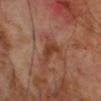<lesion>
  <biopsy_status>not biopsied; imaged during a skin examination</biopsy_status>
  <lesion_size>
    <long_diameter_mm_approx>3.5</long_diameter_mm_approx>
  </lesion_size>
  <image>
    <source>total-body photography crop</source>
    <field_of_view_mm>15</field_of_view_mm>
  </image>
  <automated_metrics>
    <shape_asymmetry>0.45</shape_asymmetry>
    <cielab_L>38</cielab_L>
    <cielab_a>23</cielab_a>
    <cielab_b>32</cielab_b>
    <vs_skin_contrast_norm>8.0</vs_skin_contrast_norm>
    <border_irregularity_0_10>4.5</border_irregularity_0_10>
    <color_variation_0_10>2.0</color_variation_0_10>
    <peripheral_color_asymmetry>1.0</peripheral_color_asymmetry>
  </automated_metrics>
  <site>arm</site>
  <lighting>cross-polarized</lighting>
  <patient>
    <sex>male</sex>
    <age_approx>70</age_approx>
  </patient>
</lesion>